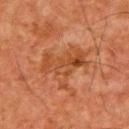biopsy_status: not biopsied; imaged during a skin examination
image:
  source: total-body photography crop
  field_of_view_mm: 15
patient:
  sex: male
  age_approx: 65
lighting: cross-polarized
lesion_size:
  long_diameter_mm_approx: 6.0
site: upper back